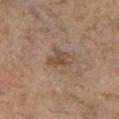follow-up — total-body-photography surveillance lesion; no biopsy
image source — total-body-photography crop, ~15 mm field of view
lighting — white-light
automated lesion analysis — an eccentricity of roughly 0.65 and a symmetry-axis asymmetry near 0.45; an average lesion color of about L≈49 a*≈16 b*≈28 (CIELAB), about 8 CIELAB-L* units darker than the surrounding skin, and a lesion-to-skin contrast of about 6.5 (normalized; higher = more distinct); lesion-presence confidence of about 100/100
anatomic site — the right upper arm
patient — female, roughly 65 years of age
lesion size — about 3 mm